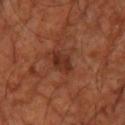biopsy_status: not biopsied; imaged during a skin examination
site: right leg
lighting: cross-polarized
image:
  source: total-body photography crop
  field_of_view_mm: 15
patient:
  sex: male
  age_approx: 60